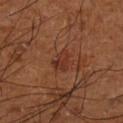Q: Was this lesion biopsied?
A: imaged on a skin check; not biopsied
Q: Where on the body is the lesion?
A: the left lower leg
Q: Patient demographics?
A: male, in their mid-60s
Q: How was the tile lit?
A: cross-polarized illumination
Q: What is the imaging modality?
A: ~15 mm crop, total-body skin-cancer survey
Q: How large is the lesion?
A: ~3 mm (longest diameter)